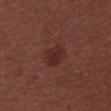follow-up — imaged on a skin check; not biopsied | lighting — white-light | patient — male, aged 28 to 32 | automated lesion analysis — a mean CIELAB color near L≈28 a*≈22 b*≈23, a lesion–skin lightness drop of about 6, and a normalized lesion–skin contrast near 6.5; border irregularity of about 2 on a 0–10 scale, a within-lesion color-variation index near 2.5/10, and peripheral color asymmetry of about 1 | lesion size — about 3 mm | imaging modality — ~15 mm tile from a whole-body skin photo | location — the upper back.